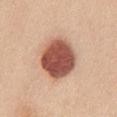Captured during whole-body skin photography for melanoma surveillance; the lesion was not biopsied.
The tile uses white-light illumination.
The lesion is located on the chest.
Approximately 6.5 mm at its widest.
A roughly 15 mm field-of-view crop from a total-body skin photograph.
A female patient, approximately 30 years of age.